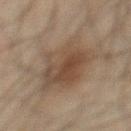Q: Was a biopsy performed?
A: imaged on a skin check; not biopsied
Q: Where on the body is the lesion?
A: the chest
Q: What did automated image analysis measure?
A: a lesion area of about 25 mm², an eccentricity of roughly 0.65, and two-axis asymmetry of about 0.3; border irregularity of about 4 on a 0–10 scale, a within-lesion color-variation index near 4.5/10, and radial color variation of about 1.5; an automated nevus-likeness rating near 55 out of 100 and a lesion-detection confidence of about 100/100
Q: Who is the patient?
A: male, aged approximately 45
Q: What is the imaging modality?
A: ~15 mm crop, total-body skin-cancer survey
Q: Illumination type?
A: cross-polarized
Q: Lesion size?
A: ~6.5 mm (longest diameter)